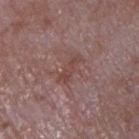biopsy status: total-body-photography surveillance lesion; no biopsy | acquisition: 15 mm crop, total-body photography | location: the chest | subject: male, aged around 65.